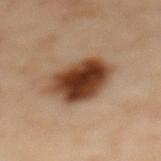Impression: Imaged during a routine full-body skin examination; the lesion was not biopsied and no histopathology is available. Image and clinical context: The recorded lesion diameter is about 6.5 mm. On the mid back. A female patient, in their 60s. This is a cross-polarized tile. Automated tile analysis of the lesion measured an area of roughly 20 mm², an eccentricity of roughly 0.7, and a symmetry-axis asymmetry near 0.15. The software also gave a border-irregularity index near 2/10, a color-variation rating of about 8.5/10, and a peripheral color-asymmetry measure near 2.5. A 15 mm crop from a total-body photograph taken for skin-cancer surveillance.